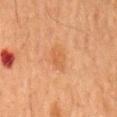Part of a total-body skin-imaging series; this lesion was reviewed on a skin check and was not flagged for biopsy.
The total-body-photography lesion software estimated a shape eccentricity near 0.8 and a shape-asymmetry score of about 0.3 (0 = symmetric). And it measured a border-irregularity index near 2.5/10, internal color variation of about 1.5 on a 0–10 scale, and a peripheral color-asymmetry measure near 0.5. The analysis additionally found a nevus-likeness score of about 5/100.
A male subject, in their mid- to late 60s.
This is a cross-polarized tile.
From the mid back.
This image is a 15 mm lesion crop taken from a total-body photograph.
The lesion's longest dimension is about 3.5 mm.